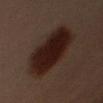follow-up = no biopsy performed (imaged during a skin exam)